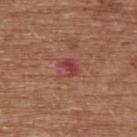Assessment:
Part of a total-body skin-imaging series; this lesion was reviewed on a skin check and was not flagged for biopsy.
Context:
From the upper back. A male subject, roughly 75 years of age. A 15 mm close-up tile from a total-body photography series done for melanoma screening. An algorithmic analysis of the crop reported an eccentricity of roughly 0.6 and two-axis asymmetry of about 0.3. The analysis additionally found an automated nevus-likeness rating near 0 out of 100 and a lesion-detection confidence of about 100/100.